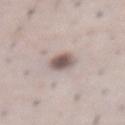Imaged during a routine full-body skin examination; the lesion was not biopsied and no histopathology is available.
The patient is a male aged 48–52.
Measured at roughly 3 mm in maximum diameter.
Cropped from a whole-body photographic skin survey; the tile spans about 15 mm.
From the abdomen.
The tile uses white-light illumination.